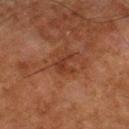Captured during whole-body skin photography for melanoma surveillance; the lesion was not biopsied.
The lesion-visualizer software estimated an area of roughly 3.5 mm², a shape eccentricity near 0.75, and a symmetry-axis asymmetry near 0.7. It also reported a normalized lesion–skin contrast near 6.5.
Longest diameter approximately 3 mm.
The lesion is on the left thigh.
Cropped from a whole-body photographic skin survey; the tile spans about 15 mm.
The patient is a male approximately 80 years of age.